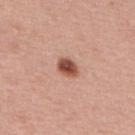biopsy_status: not biopsied; imaged during a skin examination
image:
  source: total-body photography crop
  field_of_view_mm: 15
patient:
  sex: male
  age_approx: 75
automated_metrics:
  eccentricity: 0.75
  shape_asymmetry: 0.15
  cielab_L: 51
  cielab_a: 26
  cielab_b: 29
  vs_skin_darker_L: 17.0
site: upper back
lighting: white-light
lesion_size:
  long_diameter_mm_approx: 3.0